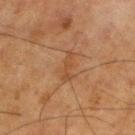| feature | finding |
|---|---|
| workup | imaged on a skin check; not biopsied |
| site | the leg |
| size | ~3.5 mm (longest diameter) |
| illumination | cross-polarized |
| image-analysis metrics | an average lesion color of about L≈37 a*≈17 b*≈29 (CIELAB), about 5 CIELAB-L* units darker than the surrounding skin, and a normalized border contrast of about 5 |
| patient | male, aged approximately 80 |
| image | 15 mm crop, total-body photography |